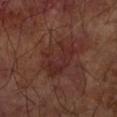| feature | finding |
|---|---|
| notes | imaged on a skin check; not biopsied |
| site | the arm |
| imaging modality | 15 mm crop, total-body photography |
| lighting | cross-polarized |
| patient | male, aged approximately 65 |